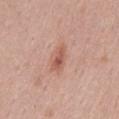Findings:
* biopsy status — imaged on a skin check; not biopsied
* imaging modality — total-body-photography crop, ~15 mm field of view
* lighting — white-light illumination
* patient — male, aged approximately 45
* site — the back
* size — ~3 mm (longest diameter)
* TBP lesion metrics — a lesion color around L≈57 a*≈24 b*≈29 in CIELAB, a lesion–skin lightness drop of about 10, and a lesion-to-skin contrast of about 7 (normalized; higher = more distinct); a border-irregularity index near 2.5/10, a color-variation rating of about 3.5/10, and a peripheral color-asymmetry measure near 1; a nevus-likeness score of about 70/100